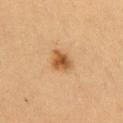biopsy_status: not biopsied; imaged during a skin examination
patient:
  sex: female
  age_approx: 40
image:
  source: total-body photography crop
  field_of_view_mm: 15
lighting: cross-polarized
site: right upper arm
automated_metrics:
  cielab_L: 47
  cielab_a: 20
  cielab_b: 36
  vs_skin_darker_L: 12.0
  vs_skin_contrast_norm: 9.0
  color_variation_0_10: 3.5